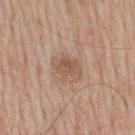Recorded during total-body skin imaging; not selected for excision or biopsy. Measured at roughly 3 mm in maximum diameter. The subject is a male aged 58 to 62. This image is a 15 mm lesion crop taken from a total-body photograph. The total-body-photography lesion software estimated roughly 9 lightness units darker than nearby skin. Located on the mid back. Imaged with white-light lighting.